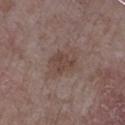biopsy status = no biopsy performed (imaged during a skin exam)
diameter = about 4 mm
anatomic site = the left upper arm
image = ~15 mm tile from a whole-body skin photo
patient = male, aged around 65
lighting = white-light illumination